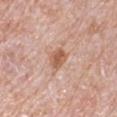No biopsy was performed on this lesion — it was imaged during a full skin examination and was not determined to be concerning. On the right upper arm. Cropped from a whole-body photographic skin survey; the tile spans about 15 mm. Approximately 3 mm at its widest. A male patient, in their 70s. The lesion-visualizer software estimated an automated nevus-likeness rating near 65 out of 100.